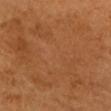workup — imaged on a skin check; not biopsied
size — ~15.5 mm (longest diameter)
illumination — cross-polarized illumination
image source — 15 mm crop, total-body photography
anatomic site — the head or neck
TBP lesion metrics — an eccentricity of roughly 0.6 and two-axis asymmetry of about 0.3; an average lesion color of about L≈49 a*≈23 b*≈38 (CIELAB), roughly 4 lightness units darker than nearby skin, and a normalized border contrast of about 3; a border-irregularity rating of about 7.5/10 and a peripheral color-asymmetry measure near 1.5
patient — female, in their 60s